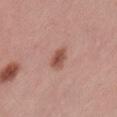Notes:
– notes · total-body-photography surveillance lesion; no biopsy
– lesion diameter · about 3 mm
– image · ~15 mm tile from a whole-body skin photo
– patient · female, approximately 55 years of age
– tile lighting · white-light
– location · the lower back